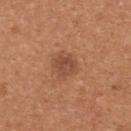| feature | finding |
|---|---|
| follow-up | total-body-photography surveillance lesion; no biopsy |
| TBP lesion metrics | an average lesion color of about L≈48 a*≈24 b*≈32 (CIELAB) and a lesion-to-skin contrast of about 6.5 (normalized; higher = more distinct); border irregularity of about 2 on a 0–10 scale, a color-variation rating of about 3/10, and radial color variation of about 1 |
| illumination | white-light |
| site | the upper back |
| patient | female, in their mid- to late 30s |
| image source | ~15 mm crop, total-body skin-cancer survey |